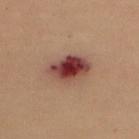Captured during whole-body skin photography for melanoma surveillance; the lesion was not biopsied. The lesion is located on the chest. A female patient, aged approximately 50. A roughly 15 mm field-of-view crop from a total-body skin photograph. About 5.5 mm across.